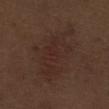Q: Was this lesion biopsied?
A: total-body-photography surveillance lesion; no biopsy